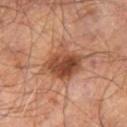{"biopsy_status": "not biopsied; imaged during a skin examination", "patient": {"sex": "male", "age_approx": 55}, "site": "leg", "automated_metrics": {"area_mm2_approx": 15.0, "eccentricity": 0.55, "shape_asymmetry": 0.2}, "image": {"source": "total-body photography crop", "field_of_view_mm": 15}, "lighting": "cross-polarized"}